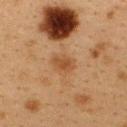Findings:
– subject: female, aged approximately 40
– acquisition: ~15 mm tile from a whole-body skin photo
– location: the upper back
– image-analysis metrics: border irregularity of about 2 on a 0–10 scale, a color-variation rating of about 1.5/10, and a peripheral color-asymmetry measure near 0.5; an automated nevus-likeness rating near 20 out of 100
– diameter: about 3 mm